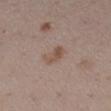follow-up = catalogued during a skin exam; not biopsied
lighting = white-light illumination
location = the right lower leg
imaging modality = ~15 mm crop, total-body skin-cancer survey
diameter = about 3 mm
patient = female, approximately 30 years of age